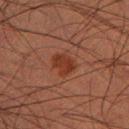Assessment: Captured during whole-body skin photography for melanoma surveillance; the lesion was not biopsied. Acquisition and patient details: Captured under cross-polarized illumination. Cropped from a whole-body photographic skin survey; the tile spans about 15 mm. A male patient, about 50 years old. The lesion-visualizer software estimated a lesion color around L≈27 a*≈22 b*≈25 in CIELAB, roughly 7 lightness units darker than nearby skin, and a normalized border contrast of about 8. It also reported a nevus-likeness score of about 65/100 and a lesion-detection confidence of about 100/100. Measured at roughly 2.5 mm in maximum diameter. From the left thigh.